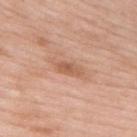Imaged during a routine full-body skin examination; the lesion was not biopsied and no histopathology is available. The lesion is on the upper back. A male patient, aged 53 to 57. A roughly 15 mm field-of-view crop from a total-body skin photograph.